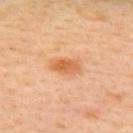Q: How large is the lesion?
A: ~3.5 mm (longest diameter)
Q: Patient demographics?
A: female, roughly 40 years of age
Q: What kind of image is this?
A: total-body-photography crop, ~15 mm field of view
Q: What lighting was used for the tile?
A: cross-polarized
Q: What is the anatomic site?
A: the upper back
Q: Automated lesion metrics?
A: an average lesion color of about L≈63 a*≈25 b*≈43 (CIELAB), roughly 11 lightness units darker than nearby skin, and a lesion-to-skin contrast of about 7.5 (normalized; higher = more distinct)